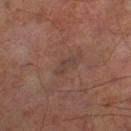| field | value |
|---|---|
| biopsy status | imaged on a skin check; not biopsied |
| automated lesion analysis | a lesion color around L≈37 a*≈16 b*≈21 in CIELAB; a border-irregularity index near 5.5/10, a within-lesion color-variation index near 0/10, and radial color variation of about 0; a nevus-likeness score of about 0/100 |
| patient | male, aged 63–67 |
| lesion size | about 2.5 mm |
| imaging modality | ~15 mm tile from a whole-body skin photo |
| body site | the leg |